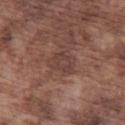- workup · imaged on a skin check; not biopsied
- imaging modality · total-body-photography crop, ~15 mm field of view
- lesion diameter · ~2.5 mm (longest diameter)
- body site · the leg
- patient · male, roughly 75 years of age
- lighting · white-light
- TBP lesion metrics · an area of roughly 5 mm² and a shape-asymmetry score of about 0.3 (0 = symmetric); a normalized border contrast of about 5; a border-irregularity index near 2.5/10, a color-variation rating of about 1.5/10, and a peripheral color-asymmetry measure near 0.5